A 15 mm close-up tile from a total-body photography series done for melanoma screening; on the left forearm; captured under white-light illumination; the subject is a male in their 40s: 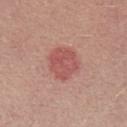Diagnosis: Biopsy histopathology demonstrated an atypical melanocytic neoplasm, classified as a lesion of indeterminate malignant potential.